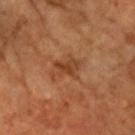The lesion was tiled from a total-body skin photograph and was not biopsied.
A female subject, approximately 70 years of age.
A region of skin cropped from a whole-body photographic capture, roughly 15 mm wide.
The lesion is on the arm.
Longest diameter approximately 3.5 mm.